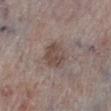Notes:
- follow-up: imaged on a skin check; not biopsied
- diameter: ≈3.5 mm
- tile lighting: white-light illumination
- location: the leg
- image source: total-body-photography crop, ~15 mm field of view
- subject: male, aged 78 to 82